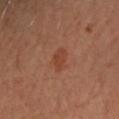Acquisition and patient details:
Cropped from a total-body skin-imaging series; the visible field is about 15 mm. On the left forearm. The lesion's longest dimension is about 2.5 mm. Imaged with cross-polarized lighting. A female patient aged 38 to 42.